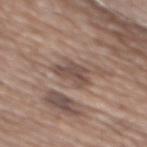Captured during whole-body skin photography for melanoma surveillance; the lesion was not biopsied. A male patient approximately 75 years of age. A lesion tile, about 15 mm wide, cut from a 3D total-body photograph. From the mid back.